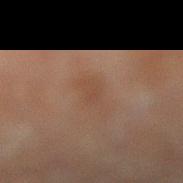This lesion was catalogued during total-body skin photography and was not selected for biopsy. The recorded lesion diameter is about 2.5 mm. A male subject, aged around 70. Automated image analysis of the tile measured an area of roughly 3 mm² and two-axis asymmetry of about 0.35. The software also gave a lesion color around L≈36 a*≈16 b*≈24 in CIELAB, about 4 CIELAB-L* units darker than the surrounding skin, and a normalized border contrast of about 4.5. It also reported a border-irregularity rating of about 3.5/10 and radial color variation of about 0. The lesion is on the right lower leg. A region of skin cropped from a whole-body photographic capture, roughly 15 mm wide.